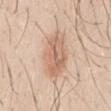| key | value |
|---|---|
| workup | total-body-photography surveillance lesion; no biopsy |
| size | about 6 mm |
| body site | the abdomen |
| acquisition | 15 mm crop, total-body photography |
| subject | male, aged 28–32 |
| lighting | white-light illumination |
| automated lesion analysis | a classifier nevus-likeness of about 70/100 |